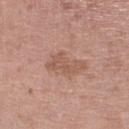The tile uses white-light illumination. A female subject, in their mid- to late 70s. The lesion's longest dimension is about 4.5 mm. A 15 mm close-up tile from a total-body photography series done for melanoma screening. Located on the left lower leg.